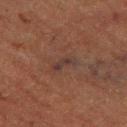No biopsy was performed on this lesion — it was imaged during a full skin examination and was not determined to be concerning. The lesion-visualizer software estimated a lesion–skin lightness drop of about 5 and a lesion-to-skin contrast of about 7 (normalized; higher = more distinct). The software also gave a border-irregularity rating of about 5/10, a within-lesion color-variation index near 0/10, and radial color variation of about 0. The software also gave an automated nevus-likeness rating near 0 out of 100. A male patient, in their mid-70s. Located on the leg. A region of skin cropped from a whole-body photographic capture, roughly 15 mm wide.